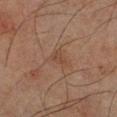{
  "biopsy_status": "not biopsied; imaged during a skin examination",
  "automated_metrics": {
    "eccentricity": 0.8,
    "shape_asymmetry": 0.4,
    "border_irregularity_0_10": 3.5,
    "color_variation_0_10": 1.0,
    "peripheral_color_asymmetry": 0.5
  },
  "patient": {
    "sex": "male",
    "age_approx": 65
  },
  "image": {
    "source": "total-body photography crop",
    "field_of_view_mm": 15
  },
  "site": "right lower leg",
  "lesion_size": {
    "long_diameter_mm_approx": 2.5
  }
}